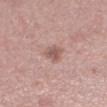Captured during whole-body skin photography for melanoma surveillance; the lesion was not biopsied. Imaged with white-light lighting. A 15 mm close-up tile from a total-body photography series done for melanoma screening. Measured at roughly 2.5 mm in maximum diameter. The subject is a female about 40 years old. The lesion is on the left lower leg.